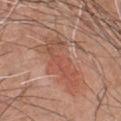{
  "biopsy_status": "not biopsied; imaged during a skin examination",
  "site": "chest",
  "patient": {
    "sex": "male",
    "age_approx": 60
  },
  "lesion_size": {
    "long_diameter_mm_approx": 6.5
  },
  "image": {
    "source": "total-body photography crop",
    "field_of_view_mm": 15
  }
}